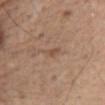Imaged during a routine full-body skin examination; the lesion was not biopsied and no histopathology is available.
A male patient aged 63–67.
A lesion tile, about 15 mm wide, cut from a 3D total-body photograph.
Located on the abdomen.